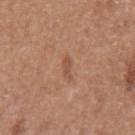Q: Was a biopsy performed?
A: total-body-photography surveillance lesion; no biopsy
Q: Lesion size?
A: ~2.5 mm (longest diameter)
Q: What are the patient's age and sex?
A: female, aged around 50
Q: Lesion location?
A: the arm
Q: How was the tile lit?
A: white-light illumination
Q: What kind of image is this?
A: 15 mm crop, total-body photography